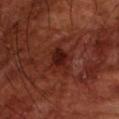Part of a total-body skin-imaging series; this lesion was reviewed on a skin check and was not flagged for biopsy. Located on the left forearm. This is a cross-polarized tile. A roughly 15 mm field-of-view crop from a total-body skin photograph. Approximately 3 mm at its widest. The subject is a male approximately 70 years of age.